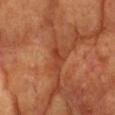follow-up = imaged on a skin check; not biopsied
site = the head or neck
subject = female, aged 73 to 77
TBP lesion metrics = an area of roughly 3 mm² and two-axis asymmetry of about 0.45
image = ~15 mm crop, total-body skin-cancer survey
illumination = cross-polarized illumination